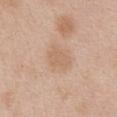– follow-up — imaged on a skin check; not biopsied
– image source — total-body-photography crop, ~15 mm field of view
– body site — the front of the torso
– subject — female, about 40 years old